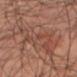The lesion was tiled from a total-body skin photograph and was not biopsied. The tile uses cross-polarized illumination. Longest diameter approximately 11.5 mm. This image is a 15 mm lesion crop taken from a total-body photograph. The lesion is located on the right thigh. The subject is a male in their mid-60s.